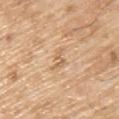<record>
<biopsy_status>not biopsied; imaged during a skin examination</biopsy_status>
<patient>
  <sex>male</sex>
  <age_approx>70</age_approx>
</patient>
<image>
  <source>total-body photography crop</source>
  <field_of_view_mm>15</field_of_view_mm>
</image>
<lesion_size>
  <long_diameter_mm_approx>3.0</long_diameter_mm_approx>
</lesion_size>
<lighting>white-light</lighting>
<site>upper back</site>
</record>